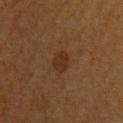Q: Is there a histopathology result?
A: catalogued during a skin exam; not biopsied
Q: How was the tile lit?
A: cross-polarized
Q: How was this image acquired?
A: ~15 mm crop, total-body skin-cancer survey
Q: Patient demographics?
A: male, approximately 55 years of age
Q: What did automated image analysis measure?
A: a mean CIELAB color near L≈26 a*≈18 b*≈28, a lesion–skin lightness drop of about 6, and a normalized lesion–skin contrast near 6.5; a peripheral color-asymmetry measure near 0.5; a classifier nevus-likeness of about 75/100 and a lesion-detection confidence of about 100/100
Q: Lesion location?
A: the upper back